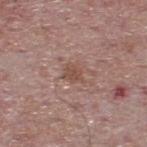<record>
<biopsy_status>not biopsied; imaged during a skin examination</biopsy_status>
<lighting>white-light</lighting>
<image>
  <source>total-body photography crop</source>
  <field_of_view_mm>15</field_of_view_mm>
</image>
<site>upper back</site>
<patient>
  <sex>male</sex>
  <age_approx>65</age_approx>
</patient>
<automated_metrics>
  <cielab_L>49</cielab_L>
  <cielab_a>20</cielab_a>
  <cielab_b>26</cielab_b>
  <vs_skin_darker_L>8.0</vs_skin_darker_L>
  <vs_skin_contrast_norm>6.5</vs_skin_contrast_norm>
</automated_metrics>
</record>